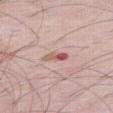Part of a total-body skin-imaging series; this lesion was reviewed on a skin check and was not flagged for biopsy.
A roughly 15 mm field-of-view crop from a total-body skin photograph.
A male patient about 65 years old.
The lesion is located on the right thigh.
Captured under white-light illumination.